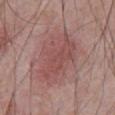Context: A 15 mm close-up tile from a total-body photography series done for melanoma screening. An algorithmic analysis of the crop reported a lesion area of about 24 mm², an eccentricity of roughly 0.8, and two-axis asymmetry of about 0.3. About 7.5 mm across. A male subject, approximately 70 years of age. Imaged with white-light lighting. On the abdomen.